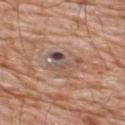Impression:
The lesion was tiled from a total-body skin photograph and was not biopsied.
Clinical summary:
The tile uses white-light illumination. The lesion is located on the upper back. About 4 mm across. A male patient about 80 years old. A roughly 15 mm field-of-view crop from a total-body skin photograph. An algorithmic analysis of the crop reported an eccentricity of roughly 0.75 and a shape-asymmetry score of about 0.55 (0 = symmetric).